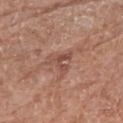Findings:
– workup: total-body-photography surveillance lesion; no biopsy
– lesion size: ~3 mm (longest diameter)
– illumination: white-light illumination
– image: ~15 mm tile from a whole-body skin photo
– patient: female, in their mid- to late 70s
– image-analysis metrics: a mean CIELAB color near L≈49 a*≈23 b*≈27 and a lesion-to-skin contrast of about 6 (normalized; higher = more distinct); border irregularity of about 8 on a 0–10 scale, a color-variation rating of about 0/10, and radial color variation of about 0
– anatomic site: the right upper arm